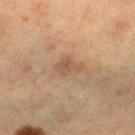Findings:
* follow-up: catalogued during a skin exam; not biopsied
* lesion diameter: ~3 mm (longest diameter)
* body site: the left lower leg
* acquisition: total-body-photography crop, ~15 mm field of view
* subject: female, aged 53–57